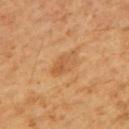Background:
The total-body-photography lesion software estimated an average lesion color of about L≈55 a*≈22 b*≈40 (CIELAB), a lesion–skin lightness drop of about 7, and a lesion-to-skin contrast of about 5 (normalized; higher = more distinct). The software also gave a border-irregularity index near 2.5/10 and internal color variation of about 3.5 on a 0–10 scale. It also reported lesion-presence confidence of about 100/100. Measured at roughly 4 mm in maximum diameter. A male subject, roughly 60 years of age. This image is a 15 mm lesion crop taken from a total-body photograph. The lesion is located on the upper back.